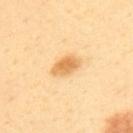{
  "biopsy_status": "not biopsied; imaged during a skin examination",
  "lighting": "cross-polarized",
  "patient": {
    "sex": "male",
    "age_approx": 40
  },
  "lesion_size": {
    "long_diameter_mm_approx": 3.5
  },
  "image": {
    "source": "total-body photography crop",
    "field_of_view_mm": 15
  },
  "automated_metrics": {
    "eccentricity": 0.85,
    "cielab_L": 72,
    "cielab_a": 21,
    "cielab_b": 47,
    "nevus_likeness_0_100": 95,
    "lesion_detection_confidence_0_100": 100
  },
  "site": "upper back"
}